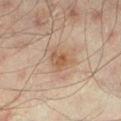Q: Was this lesion biopsied?
A: imaged on a skin check; not biopsied
Q: What kind of image is this?
A: total-body-photography crop, ~15 mm field of view
Q: Lesion location?
A: the left lower leg
Q: Who is the patient?
A: male, aged 43–47
Q: What is the lesion's diameter?
A: about 3 mm
Q: What did automated image analysis measure?
A: an automated nevus-likeness rating near 45 out of 100